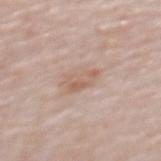{
  "lighting": "white-light",
  "patient": {
    "sex": "female",
    "age_approx": 65
  },
  "image": {
    "source": "total-body photography crop",
    "field_of_view_mm": 15
  },
  "site": "upper back"
}